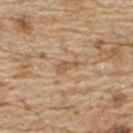Q: Was a biopsy performed?
A: catalogued during a skin exam; not biopsied
Q: What is the imaging modality?
A: total-body-photography crop, ~15 mm field of view
Q: How was the tile lit?
A: white-light
Q: What is the anatomic site?
A: the upper back
Q: What are the patient's age and sex?
A: male, aged 68 to 72
Q: Automated lesion metrics?
A: a border-irregularity index near 4.5/10 and a color-variation rating of about 0.5/10